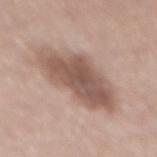Q: Was this lesion biopsied?
A: no biopsy performed (imaged during a skin exam)
Q: How was this image acquired?
A: ~15 mm tile from a whole-body skin photo
Q: What is the lesion's diameter?
A: ~8.5 mm (longest diameter)
Q: Where on the body is the lesion?
A: the mid back
Q: Who is the patient?
A: female, approximately 60 years of age